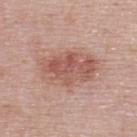* notes · total-body-photography surveillance lesion; no biopsy
* lesion diameter · ~7 mm (longest diameter)
* image source · ~15 mm crop, total-body skin-cancer survey
* subject · female, in their 50s
* site · the upper back
* tile lighting · white-light illumination